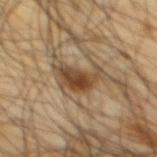Captured during whole-body skin photography for melanoma surveillance; the lesion was not biopsied. A male patient aged 63–67. A 15 mm close-up extracted from a 3D total-body photography capture. The lesion is on the mid back.